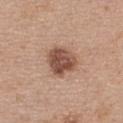Imaged during a routine full-body skin examination; the lesion was not biopsied and no histopathology is available. On the chest. This is a white-light tile. A female subject roughly 35 years of age. A 15 mm crop from a total-body photograph taken for skin-cancer surveillance. The lesion-visualizer software estimated a mean CIELAB color near L≈51 a*≈21 b*≈28 and roughly 14 lightness units darker than nearby skin. The analysis additionally found a classifier nevus-likeness of about 65/100 and lesion-presence confidence of about 100/100. The lesion's longest dimension is about 4 mm.